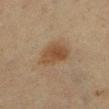notes=imaged on a skin check; not biopsied
illumination=cross-polarized
subject=female, approximately 55 years of age
lesion diameter=about 4.5 mm
image source=~15 mm crop, total-body skin-cancer survey
site=the left lower leg